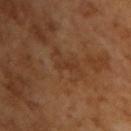{"biopsy_status": "not biopsied; imaged during a skin examination", "lesion_size": {"long_diameter_mm_approx": 2.5}, "patient": {"sex": "male", "age_approx": 65}, "lighting": "cross-polarized", "image": {"source": "total-body photography crop", "field_of_view_mm": 15}}